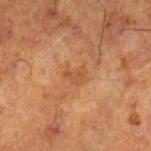follow-up: no biopsy performed (imaged during a skin exam); lesion diameter: ≈2.5 mm; image: 15 mm crop, total-body photography; body site: the leg; illumination: cross-polarized illumination; subject: male, in their 70s.